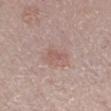Image and clinical context: Measured at roughly 2.5 mm in maximum diameter. An algorithmic analysis of the crop reported an area of roughly 3.5 mm², an eccentricity of roughly 0.8, and a symmetry-axis asymmetry near 0.4. The software also gave a mean CIELAB color near L≈57 a*≈19 b*≈23 and a normalized lesion–skin contrast near 5. A 15 mm crop from a total-body photograph taken for skin-cancer surveillance. The tile uses white-light illumination. The subject is a female in their mid-60s. The lesion is located on the leg.